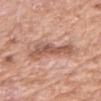{"biopsy_status": "not biopsied; imaged during a skin examination", "image": {"source": "total-body photography crop", "field_of_view_mm": 15}, "lesion_size": {"long_diameter_mm_approx": 5.0}, "site": "right upper arm", "patient": {"sex": "female", "age_approx": 75}}